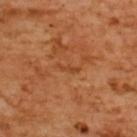This lesion was catalogued during total-body skin photography and was not selected for biopsy. The total-body-photography lesion software estimated a lesion–skin lightness drop of about 6. It also reported border irregularity of about 5 on a 0–10 scale and peripheral color asymmetry of about 0. The lesion is on the back. Cropped from a total-body skin-imaging series; the visible field is about 15 mm. The patient is a female approximately 55 years of age.